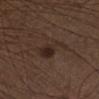From the left lower leg. The patient is a male approximately 50 years of age. This image is a 15 mm lesion crop taken from a total-body photograph.